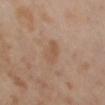biopsy status = imaged on a skin check; not biopsied | image source = total-body-photography crop, ~15 mm field of view | lesion size = ≈3 mm | subject = female, aged 53–57 | lighting = cross-polarized | anatomic site = the lower back.